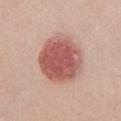No biopsy was performed on this lesion — it was imaged during a full skin examination and was not determined to be concerning. This is a white-light tile. A region of skin cropped from a whole-body photographic capture, roughly 15 mm wide. The subject is a female aged 58 to 62. Approximately 6 mm at its widest. From the chest. The lesion-visualizer software estimated a footprint of about 25 mm², a shape eccentricity near 0.6, and two-axis asymmetry of about 0.1. The software also gave a nevus-likeness score of about 100/100.